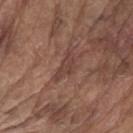The lesion was photographed on a routine skin check and not biopsied; there is no pathology result.
A 15 mm close-up tile from a total-body photography series done for melanoma screening.
A male patient, in their 80s.
Automated image analysis of the tile measured a footprint of about 5 mm² and an outline eccentricity of about 0.85 (0 = round, 1 = elongated). It also reported a mean CIELAB color near L≈41 a*≈20 b*≈24, roughly 7 lightness units darker than nearby skin, and a normalized border contrast of about 6. The analysis additionally found border irregularity of about 5 on a 0–10 scale and a peripheral color-asymmetry measure near 0.5.
On the left upper arm.